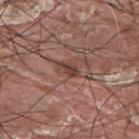| field | value |
|---|---|
| notes | catalogued during a skin exam; not biopsied |
| anatomic site | the upper back |
| subject | male, aged 38–42 |
| acquisition | total-body-photography crop, ~15 mm field of view |